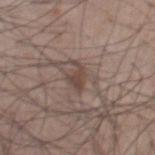workup = no biopsy performed (imaged during a skin exam) | patient = male, aged 53 to 57 | acquisition = ~15 mm crop, total-body skin-cancer survey | diameter = ~3.5 mm (longest diameter) | body site = the chest | TBP lesion metrics = an area of roughly 4 mm² and an eccentricity of roughly 0.85; a border-irregularity index near 5/10 and a peripheral color-asymmetry measure near 0.5; an automated nevus-likeness rating near 5 out of 100 and a lesion-detection confidence of about 100/100 | illumination = white-light.